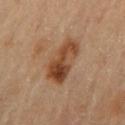The tile uses cross-polarized illumination. Cropped from a whole-body photographic skin survey; the tile spans about 15 mm. A female subject aged approximately 65. The lesion is located on the arm.